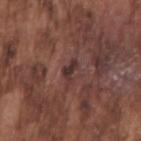biopsy_status: not biopsied; imaged during a skin examination
lighting: white-light
lesion_size:
  long_diameter_mm_approx: 2.5
image:
  source: total-body photography crop
  field_of_view_mm: 15
site: right upper arm
patient:
  sex: male
  age_approx: 75